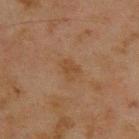| key | value |
|---|---|
| follow-up | no biopsy performed (imaged during a skin exam) |
| anatomic site | the upper back |
| subject | male, aged 43 to 47 |
| diameter | about 2.5 mm |
| image source | ~15 mm crop, total-body skin-cancer survey |
| automated metrics | a mean CIELAB color near L≈37 a*≈16 b*≈29, a lesion–skin lightness drop of about 5, and a normalized border contrast of about 5.5; a within-lesion color-variation index near 1.5/10 and a peripheral color-asymmetry measure near 0.5; a classifier nevus-likeness of about 0/100 and lesion-presence confidence of about 100/100 |